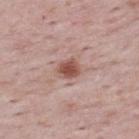<case>
<lighting>white-light</lighting>
<site>upper back</site>
<image>
  <source>total-body photography crop</source>
  <field_of_view_mm>15</field_of_view_mm>
</image>
<patient>
  <sex>female</sex>
  <age_approx>50</age_approx>
</patient>
<lesion_size>
  <long_diameter_mm_approx>2.5</long_diameter_mm_approx>
</lesion_size>
</case>